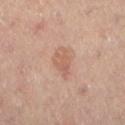Case summary:
- biopsy status · no biopsy performed (imaged during a skin exam)